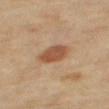site: right thigh
lesion_size:
  long_diameter_mm_approx: 4.5
automated_metrics:
  cielab_L: 47
  cielab_a: 19
  cielab_b: 30
  vs_skin_darker_L: 10.0
  vs_skin_contrast_norm: 8.0
image:
  source: total-body photography crop
  field_of_view_mm: 15
lighting: cross-polarized
patient:
  sex: female
  age_approx: 60